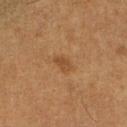subject: male, roughly 55 years of age
acquisition: ~15 mm crop, total-body skin-cancer survey
tile lighting: cross-polarized
diameter: ≈2.5 mm
automated metrics: a footprint of about 3 mm², a shape eccentricity near 0.85, and a shape-asymmetry score of about 0.3 (0 = symmetric); an average lesion color of about L≈41 a*≈19 b*≈33 (CIELAB) and a normalized border contrast of about 6; a border-irregularity index near 2.5/10 and radial color variation of about 0.5; a lesion-detection confidence of about 100/100
site: the right lower leg